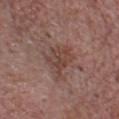{"biopsy_status": "not biopsied; imaged during a skin examination", "site": "chest", "image": {"source": "total-body photography crop", "field_of_view_mm": 15}, "patient": {"sex": "male", "age_approx": 65}}